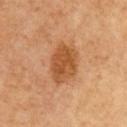Findings:
- patient: male, in their mid- to late 60s
- lesion size: ≈4.5 mm
- lighting: cross-polarized
- acquisition: total-body-photography crop, ~15 mm field of view